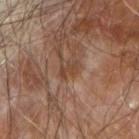{"biopsy_status": "not biopsied; imaged during a skin examination", "patient": {"sex": "male", "age_approx": 70}, "automated_metrics": {"area_mm2_approx": 3.0, "eccentricity": 0.85, "shape_asymmetry": 0.4, "nevus_likeness_0_100": 0, "lesion_detection_confidence_0_100": 90}, "lighting": "cross-polarized", "image": {"source": "total-body photography crop", "field_of_view_mm": 15}, "lesion_size": {"long_diameter_mm_approx": 2.5}, "site": "right forearm"}